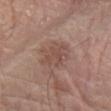Assessment: Imaged during a routine full-body skin examination; the lesion was not biopsied and no histopathology is available. Clinical summary: The subject is a male aged approximately 80. On the arm. A region of skin cropped from a whole-body photographic capture, roughly 15 mm wide.